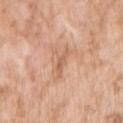{
  "biopsy_status": "not biopsied; imaged during a skin examination",
  "lesion_size": {
    "long_diameter_mm_approx": 2.5
  },
  "patient": {
    "sex": "female",
    "age_approx": 75
  },
  "site": "right upper arm",
  "lighting": "white-light",
  "image": {
    "source": "total-body photography crop",
    "field_of_view_mm": 15
  }
}